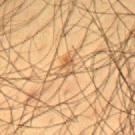This lesion was catalogued during total-body skin photography and was not selected for biopsy. Longest diameter approximately 2.5 mm. On the back. An algorithmic analysis of the crop reported a footprint of about 3.5 mm² and a shape-asymmetry score of about 0.45 (0 = symmetric). And it measured a lesion color around L≈47 a*≈16 b*≈33 in CIELAB, about 8 CIELAB-L* units darker than the surrounding skin, and a normalized border contrast of about 6.5. It also reported a detector confidence of about 80 out of 100 that the crop contains a lesion. A 15 mm close-up tile from a total-body photography series done for melanoma screening. A male patient, aged 58 to 62. The tile uses cross-polarized illumination.